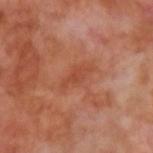• notes · no biopsy performed (imaged during a skin exam)
• anatomic site · the left upper arm
• tile lighting · cross-polarized illumination
• patient · male, about 70 years old
• size · about 3.5 mm
• automated metrics · a mean CIELAB color near L≈46 a*≈28 b*≈33, a lesion–skin lightness drop of about 6, and a lesion-to-skin contrast of about 5 (normalized; higher = more distinct)
• imaging modality · ~15 mm tile from a whole-body skin photo